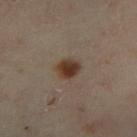{
  "biopsy_status": "not biopsied; imaged during a skin examination",
  "site": "right lower leg",
  "patient": {
    "sex": "female",
    "age_approx": 35
  },
  "lighting": "cross-polarized",
  "lesion_size": {
    "long_diameter_mm_approx": 2.5
  },
  "automated_metrics": {
    "area_mm2_approx": 5.0,
    "eccentricity": 0.55,
    "shape_asymmetry": 0.25,
    "cielab_L": 34,
    "cielab_a": 15,
    "cielab_b": 25,
    "vs_skin_darker_L": 12.0,
    "vs_skin_contrast_norm": 11.5,
    "lesion_detection_confidence_0_100": 100
  },
  "image": {
    "source": "total-body photography crop",
    "field_of_view_mm": 15
  }
}